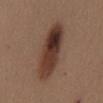| feature | finding |
|---|---|
| image source | ~15 mm tile from a whole-body skin photo |
| illumination | white-light |
| subject | female, aged 38 to 42 |
| location | the mid back |
| size | ≈8.5 mm |
| image-analysis metrics | border irregularity of about 2.5 on a 0–10 scale, a color-variation rating of about 9.5/10, and a peripheral color-asymmetry measure near 3.5; a nevus-likeness score of about 100/100 |